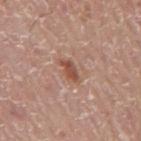Findings:
- automated lesion analysis — a mean CIELAB color near L≈51 a*≈23 b*≈29 and a lesion-to-skin contrast of about 8 (normalized; higher = more distinct)
- illumination — white-light illumination
- patient — male, in their mid- to late 70s
- anatomic site — the mid back
- imaging modality — ~15 mm tile from a whole-body skin photo